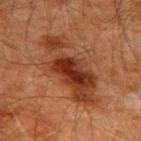{
  "biopsy_status": "not biopsied; imaged during a skin examination",
  "site": "upper back",
  "image": {
    "source": "total-body photography crop",
    "field_of_view_mm": 15
  },
  "lesion_size": {
    "long_diameter_mm_approx": 9.5
  },
  "patient": {
    "sex": "male",
    "age_approx": 60
  }
}